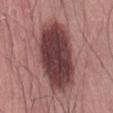Clinical impression: The lesion was photographed on a routine skin check and not biopsied; there is no pathology result. Clinical summary: The lesion is located on the abdomen. A lesion tile, about 15 mm wide, cut from a 3D total-body photograph. About 9.5 mm across. Captured under white-light illumination. A male subject aged approximately 50.